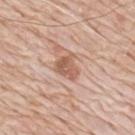The lesion was tiled from a total-body skin photograph and was not biopsied. Imaged with white-light lighting. From the back. Automated image analysis of the tile measured a mean CIELAB color near L≈58 a*≈21 b*≈29, a lesion–skin lightness drop of about 11, and a lesion-to-skin contrast of about 7.5 (normalized; higher = more distinct). And it measured a detector confidence of about 100 out of 100 that the crop contains a lesion. Cropped from a whole-body photographic skin survey; the tile spans about 15 mm. A male subject aged 78 to 82. About 3 mm across.